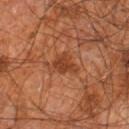Case summary:
* biopsy status — catalogued during a skin exam; not biopsied
* patient — male, aged 58–62
* location — the leg
* imaging modality — ~15 mm crop, total-body skin-cancer survey